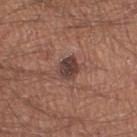Case summary:
- follow-up — total-body-photography surveillance lesion; no biopsy
- acquisition — total-body-photography crop, ~15 mm field of view
- image-analysis metrics — an average lesion color of about L≈40 a*≈19 b*≈21 (CIELAB), roughly 12 lightness units darker than nearby skin, and a normalized border contrast of about 10; a classifier nevus-likeness of about 35/100 and a lesion-detection confidence of about 100/100
- tile lighting — white-light
- site — the left thigh
- diameter — ≈3 mm
- patient — male, approximately 40 years of age